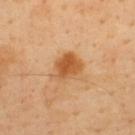- notes — no biopsy performed (imaged during a skin exam)
- image-analysis metrics — a footprint of about 8.5 mm² and a shape-asymmetry score of about 0.25 (0 = symmetric); lesion-presence confidence of about 100/100
- patient — male, approximately 40 years of age
- illumination — cross-polarized illumination
- body site — the upper back
- image — 15 mm crop, total-body photography
- lesion size — about 3.5 mm